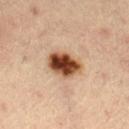Acquisition and patient details:
A male subject about 65 years old. The lesion-visualizer software estimated an area of roughly 10 mm², a shape eccentricity near 0.75, and two-axis asymmetry of about 0.15. This is a cross-polarized tile. Located on the left thigh. A 15 mm crop from a total-body photograph taken for skin-cancer surveillance. About 4.5 mm across.